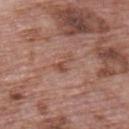automated lesion analysis = border irregularity of about 6.5 on a 0–10 scale, a color-variation rating of about 0/10, and peripheral color asymmetry of about 0; lesion-presence confidence of about 100/100 | image source = 15 mm crop, total-body photography | location = the back | subject = male, in their 70s | lesion diameter = ≈2.5 mm | lighting = white-light.